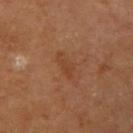biopsy status: total-body-photography surveillance lesion; no biopsy | size: about 3.5 mm | body site: the left upper arm | patient: male, aged 63–67 | image: ~15 mm crop, total-body skin-cancer survey | tile lighting: cross-polarized illumination.